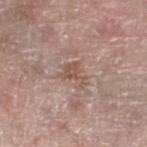biopsy status: imaged on a skin check; not biopsied
acquisition: 15 mm crop, total-body photography
patient: female, aged 58 to 62
illumination: white-light illumination
location: the right lower leg
diameter: ~3 mm (longest diameter)
automated metrics: a lesion color around L≈53 a*≈18 b*≈25 in CIELAB, roughly 8 lightness units darker than nearby skin, and a normalized lesion–skin contrast near 6.5